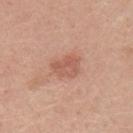Assessment:
Recorded during total-body skin imaging; not selected for excision or biopsy.
Acquisition and patient details:
Captured under white-light illumination. About 3 mm across. The patient is a male approximately 25 years of age. Cropped from a whole-body photographic skin survey; the tile spans about 15 mm. Automated image analysis of the tile measured an automated nevus-likeness rating near 10 out of 100 and lesion-presence confidence of about 100/100. The lesion is located on the left upper arm.